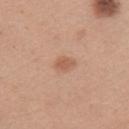No biopsy was performed on this lesion — it was imaged during a full skin examination and was not determined to be concerning. On the left upper arm. Cropped from a whole-body photographic skin survey; the tile spans about 15 mm. A male subject, in their mid-20s. Imaged with white-light lighting.